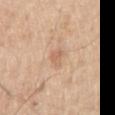{"biopsy_status": "not biopsied; imaged during a skin examination", "image": {"source": "total-body photography crop", "field_of_view_mm": 15}, "lighting": "white-light", "patient": {"sex": "male", "age_approx": 55}, "automated_metrics": {"area_mm2_approx": 3.5, "shape_asymmetry": 0.35, "border_irregularity_0_10": 4.0, "peripheral_color_asymmetry": 0.5}, "site": "right upper arm", "lesion_size": {"long_diameter_mm_approx": 2.5}}